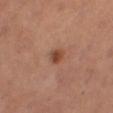Impression: Captured during whole-body skin photography for melanoma surveillance; the lesion was not biopsied. Background: The patient is a female about 55 years old. The lesion is located on the right thigh. Captured under cross-polarized illumination. Cropped from a whole-body photographic skin survey; the tile spans about 15 mm.